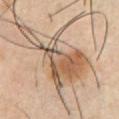workup = total-body-photography surveillance lesion; no biopsy
lesion size = ≈13.5 mm
body site = the chest
patient = male, aged around 40
image = 15 mm crop, total-body photography
tile lighting = cross-polarized illumination